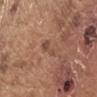Case summary:
* notes: no biopsy performed (imaged during a skin exam)
* subject: male, aged around 70
* body site: the left upper arm
* imaging modality: 15 mm crop, total-body photography
* lesion size: about 3 mm
* tile lighting: white-light
* automated lesion analysis: a lesion area of about 3 mm², an eccentricity of roughly 0.9, and a shape-asymmetry score of about 0.5 (0 = symmetric); an average lesion color of about L≈47 a*≈22 b*≈28 (CIELAB), a lesion–skin lightness drop of about 8, and a lesion-to-skin contrast of about 6 (normalized; higher = more distinct); border irregularity of about 5.5 on a 0–10 scale and a within-lesion color-variation index near 1/10